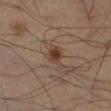Recorded during total-body skin imaging; not selected for excision or biopsy.
The lesion is located on the right lower leg.
The tile uses cross-polarized illumination.
About 3.5 mm across.
A 15 mm close-up extracted from a 3D total-body photography capture.
A male subject aged 48 to 52.